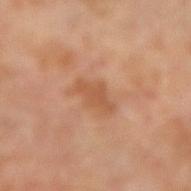Captured during whole-body skin photography for melanoma surveillance; the lesion was not biopsied. Approximately 3.5 mm at its widest. A female patient aged approximately 70. The lesion is located on the right lower leg. Captured under cross-polarized illumination. A lesion tile, about 15 mm wide, cut from a 3D total-body photograph.